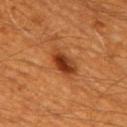notes: total-body-photography surveillance lesion; no biopsy
diameter: ≈4 mm
patient: male, aged 58 to 62
anatomic site: the mid back
tile lighting: cross-polarized
image: total-body-photography crop, ~15 mm field of view
TBP lesion metrics: an outline eccentricity of about 0.85 (0 = round, 1 = elongated); a color-variation rating of about 5.5/10 and peripheral color asymmetry of about 1.5